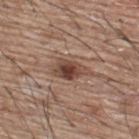| field | value |
|---|---|
| workup | catalogued during a skin exam; not biopsied |
| lesion size | ≈4.5 mm |
| subject | male, aged around 65 |
| lighting | white-light illumination |
| image | 15 mm crop, total-body photography |
| anatomic site | the upper back |